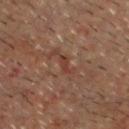Impression: Recorded during total-body skin imaging; not selected for excision or biopsy. Context: On the head or neck. Cropped from a total-body skin-imaging series; the visible field is about 15 mm. A male subject, roughly 60 years of age.